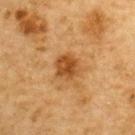Captured during whole-body skin photography for melanoma surveillance; the lesion was not biopsied.
A 15 mm crop from a total-body photograph taken for skin-cancer surveillance.
From the back.
A male subject, in their mid- to late 80s or older.